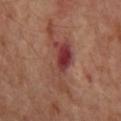notes — no biopsy performed (imaged during a skin exam).